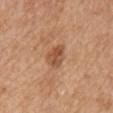Image and clinical context: On the right upper arm. Longest diameter approximately 3 mm. A male patient aged 63 to 67. A 15 mm close-up tile from a total-body photography series done for melanoma screening.